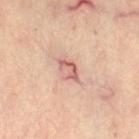biopsy status: total-body-photography surveillance lesion; no biopsy
image-analysis metrics: a footprint of about 4 mm², an outline eccentricity of about 0.85 (0 = round, 1 = elongated), and a shape-asymmetry score of about 0.45 (0 = symmetric); an average lesion color of about L≈63 a*≈26 b*≈26 (CIELAB), about 12 CIELAB-L* units darker than the surrounding skin, and a lesion-to-skin contrast of about 7.5 (normalized; higher = more distinct)
illumination: cross-polarized
patient: female, in their 40s
imaging modality: 15 mm crop, total-body photography
lesion diameter: ~3.5 mm (longest diameter)
body site: the right thigh